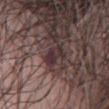Part of a total-body skin-imaging series; this lesion was reviewed on a skin check and was not flagged for biopsy. On the abdomen. Cropped from a whole-body photographic skin survey; the tile spans about 15 mm. A female patient, aged 58 to 62. Automated image analysis of the tile measured a border-irregularity index near 3/10 and peripheral color asymmetry of about 2. The tile uses white-light illumination.